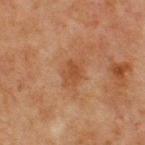notes: total-body-photography surveillance lesion; no biopsy | anatomic site: the chest | illumination: cross-polarized | patient: male, aged around 65 | lesion diameter: ≈3 mm | image source: total-body-photography crop, ~15 mm field of view.